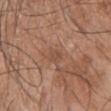Imaged during a routine full-body skin examination; the lesion was not biopsied and no histopathology is available. A male patient in their mid- to late 40s. The lesion's longest dimension is about 2.5 mm. Located on the chest. This is a white-light tile. A close-up tile cropped from a whole-body skin photograph, about 15 mm across.